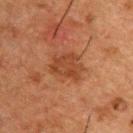  image:
    source: total-body photography crop
    field_of_view_mm: 15
  lighting: cross-polarized
  patient:
    sex: male
    age_approx: 50
  lesion_size:
    long_diameter_mm_approx: 3.5
  automated_metrics:
    area_mm2_approx: 9.0
    eccentricity: 0.55
    shape_asymmetry: 0.3
    cielab_L: 34
    cielab_a: 22
    cielab_b: 29
    vs_skin_contrast_norm: 6.5
    border_irregularity_0_10: 3.5
    color_variation_0_10: 2.5
    peripheral_color_asymmetry: 1.0
    nevus_likeness_0_100: 0
  site: upper back